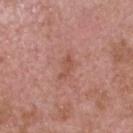Clinical impression: Recorded during total-body skin imaging; not selected for excision or biopsy.